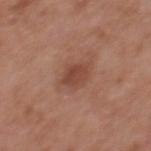notes: no biopsy performed (imaged during a skin exam) | lighting: white-light | body site: the back | size: about 3 mm | subject: male, about 70 years old | acquisition: ~15 mm tile from a whole-body skin photo.